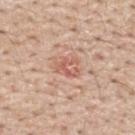follow-up: no biopsy performed (imaged during a skin exam) | anatomic site: the upper back | size: about 3 mm | subject: male, aged around 55 | automated lesion analysis: a lesion color around L≈59 a*≈25 b*≈29 in CIELAB, a lesion–skin lightness drop of about 9, and a normalized lesion–skin contrast near 6; a nevus-likeness score of about 0/100 | image source: ~15 mm crop, total-body skin-cancer survey.